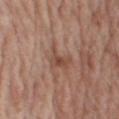follow-up: total-body-photography surveillance lesion; no biopsy | image-analysis metrics: a lesion area of about 6 mm² and a shape-asymmetry score of about 0.45 (0 = symmetric) | subject: male, aged around 70 | site: the right upper arm | image source: 15 mm crop, total-body photography.